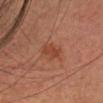| key | value |
|---|---|
| biopsy status | imaged on a skin check; not biopsied |